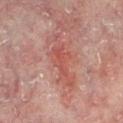follow-up=total-body-photography surveillance lesion; no biopsy
acquisition=total-body-photography crop, ~15 mm field of view
TBP lesion metrics=a mean CIELAB color near L≈47 a*≈26 b*≈25, roughly 7 lightness units darker than nearby skin, and a normalized lesion–skin contrast near 6; border irregularity of about 8.5 on a 0–10 scale, a color-variation rating of about 3/10, and radial color variation of about 1; a nevus-likeness score of about 0/100 and lesion-presence confidence of about 95/100
size=about 6.5 mm
subject=male, aged 58–62
anatomic site=the left lower leg